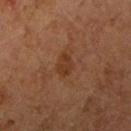No biopsy was performed on this lesion — it was imaged during a full skin examination and was not determined to be concerning. A female patient aged 58 to 62. Captured under cross-polarized illumination. Automated image analysis of the tile measured an area of roughly 4.5 mm², an outline eccentricity of about 0.75 (0 = round, 1 = elongated), and two-axis asymmetry of about 0.25. It also reported an average lesion color of about L≈31 a*≈19 b*≈28 (CIELAB), a lesion–skin lightness drop of about 6, and a lesion-to-skin contrast of about 6.5 (normalized; higher = more distinct). It also reported peripheral color asymmetry of about 0.5. The lesion is on the right upper arm. Cropped from a whole-body photographic skin survey; the tile spans about 15 mm.